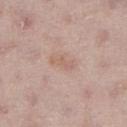Q: What kind of image is this?
A: ~15 mm tile from a whole-body skin photo
Q: Lesion location?
A: the leg
Q: Who is the patient?
A: female, about 50 years old
Q: Automated lesion metrics?
A: an average lesion color of about L≈61 a*≈18 b*≈26 (CIELAB), about 6 CIELAB-L* units darker than the surrounding skin, and a lesion-to-skin contrast of about 5 (normalized; higher = more distinct); a border-irregularity index near 5/10 and internal color variation of about 0 on a 0–10 scale; a detector confidence of about 100 out of 100 that the crop contains a lesion